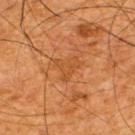notes: imaged on a skin check; not biopsied
subject: male, aged 63 to 67
diameter: ≈3 mm
lighting: cross-polarized illumination
site: the upper back
acquisition: ~15 mm crop, total-body skin-cancer survey
automated metrics: an area of roughly 5 mm²